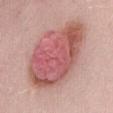The lesion was tiled from a total-body skin photograph and was not biopsied. From the abdomen. Cropped from a total-body skin-imaging series; the visible field is about 15 mm. The patient is a male aged 38–42.